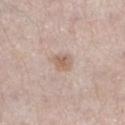Recorded during total-body skin imaging; not selected for excision or biopsy. The tile uses white-light illumination. The lesion's longest dimension is about 2.5 mm. From the left lower leg. A female subject, about 45 years old. A lesion tile, about 15 mm wide, cut from a 3D total-body photograph.